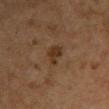notes: total-body-photography surveillance lesion; no biopsy | lesion diameter: ≈3 mm | acquisition: 15 mm crop, total-body photography | site: the left upper arm | lighting: cross-polarized illumination | patient: female, about 60 years old.